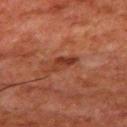Assessment: No biopsy was performed on this lesion — it was imaged during a full skin examination and was not determined to be concerning. Acquisition and patient details: A male patient approximately 80 years of age. This is a cross-polarized tile. Approximately 3.5 mm at its widest. Cropped from a total-body skin-imaging series; the visible field is about 15 mm. On the mid back.